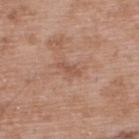Q: Was a biopsy performed?
A: catalogued during a skin exam; not biopsied
Q: Lesion size?
A: ~3 mm (longest diameter)
Q: How was the tile lit?
A: white-light illumination
Q: Where on the body is the lesion?
A: the upper back
Q: What are the patient's age and sex?
A: male, roughly 50 years of age
Q: What kind of image is this?
A: ~15 mm tile from a whole-body skin photo
Q: Automated lesion metrics?
A: a footprint of about 2.5 mm², an eccentricity of roughly 0.9, and a symmetry-axis asymmetry near 0.35; an average lesion color of about L≈53 a*≈22 b*≈29 (CIELAB), about 7 CIELAB-L* units darker than the surrounding skin, and a lesion-to-skin contrast of about 5.5 (normalized; higher = more distinct); a border-irregularity index near 4/10 and a peripheral color-asymmetry measure near 0